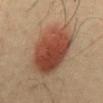Case summary:
* workup: imaged on a skin check; not biopsied
* TBP lesion metrics: an area of roughly 30 mm² and a shape eccentricity near 0.7; border irregularity of about 1.5 on a 0–10 scale and a peripheral color-asymmetry measure near 2; an automated nevus-likeness rating near 100 out of 100
* body site: the front of the torso
* illumination: cross-polarized
* image source: ~15 mm crop, total-body skin-cancer survey
* lesion size: ≈7 mm
* patient: male, approximately 40 years of age